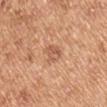notes = total-body-photography surveillance lesion; no biopsy | illumination = white-light illumination | image = ~15 mm tile from a whole-body skin photo | patient = male, aged around 55 | site = the right upper arm.